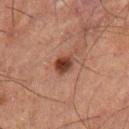| field | value |
|---|---|
| follow-up | catalogued during a skin exam; not biopsied |